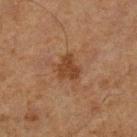Recorded during total-body skin imaging; not selected for excision or biopsy.
The lesion is located on the right lower leg.
The recorded lesion diameter is about 3 mm.
A region of skin cropped from a whole-body photographic capture, roughly 15 mm wide.
The lesion-visualizer software estimated an area of roughly 6 mm², an outline eccentricity of about 0.5 (0 = round, 1 = elongated), and a symmetry-axis asymmetry near 0.35. The software also gave a mean CIELAB color near L≈33 a*≈18 b*≈28 and a normalized border contrast of about 7.5. The analysis additionally found an automated nevus-likeness rating near 15 out of 100 and a lesion-detection confidence of about 100/100.
A male patient aged approximately 65.